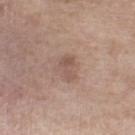lesion size = ≈3 mm
body site = the right lower leg
imaging modality = 15 mm crop, total-body photography
lighting = white-light illumination
subject = female, in their mid-50s